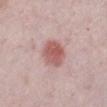{
  "biopsy_status": "not biopsied; imaged during a skin examination",
  "site": "leg",
  "patient": {
    "sex": "female",
    "age_approx": 45
  },
  "image": {
    "source": "total-body photography crop",
    "field_of_view_mm": 15
  },
  "lighting": "white-light"
}